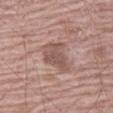Impression: This lesion was catalogued during total-body skin photography and was not selected for biopsy. Background: On the left thigh. A lesion tile, about 15 mm wide, cut from a 3D total-body photograph. An algorithmic analysis of the crop reported a footprint of about 10 mm². It also reported a mean CIELAB color near L≈53 a*≈19 b*≈24, a lesion–skin lightness drop of about 9, and a lesion-to-skin contrast of about 6.5 (normalized; higher = more distinct). And it measured border irregularity of about 3.5 on a 0–10 scale and internal color variation of about 3 on a 0–10 scale. A male patient, aged around 70. Captured under white-light illumination. The lesion's longest dimension is about 4 mm.